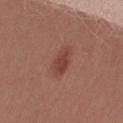  biopsy_status: not biopsied; imaged during a skin examination
  site: right thigh
  image:
    source: total-body photography crop
    field_of_view_mm: 15
  patient:
    sex: female
    age_approx: 20